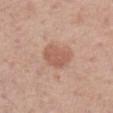Recorded during total-body skin imaging; not selected for excision or biopsy.
A male subject aged approximately 60.
A region of skin cropped from a whole-body photographic capture, roughly 15 mm wide.
Approximately 4 mm at its widest.
The lesion is located on the arm.
An algorithmic analysis of the crop reported a lesion-to-skin contrast of about 6 (normalized; higher = more distinct). The software also gave a classifier nevus-likeness of about 55/100.